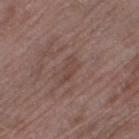Part of a total-body skin-imaging series; this lesion was reviewed on a skin check and was not flagged for biopsy.
From the left thigh.
Cropped from a total-body skin-imaging series; the visible field is about 15 mm.
Captured under white-light illumination.
A female patient about 70 years old.